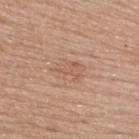Assessment:
The lesion was tiled from a total-body skin photograph and was not biopsied.
Background:
The lesion is located on the upper back. A female subject, aged 48–52. A 15 mm close-up extracted from a 3D total-body photography capture.